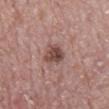The lesion was photographed on a routine skin check and not biopsied; there is no pathology result. Measured at roughly 3 mm in maximum diameter. A male subject, roughly 70 years of age. A roughly 15 mm field-of-view crop from a total-body skin photograph. Captured under white-light illumination. The lesion is on the mid back.